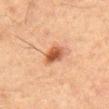This lesion was catalogued during total-body skin photography and was not selected for biopsy.